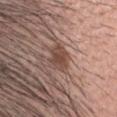biopsy status: imaged on a skin check; not biopsied | location: the head or neck | lighting: white-light | size: about 3.5 mm | automated metrics: a footprint of about 7 mm², an outline eccentricity of about 0.65 (0 = round, 1 = elongated), and a shape-asymmetry score of about 0.2 (0 = symmetric); a lesion color around L≈46 a*≈19 b*≈25 in CIELAB, roughly 10 lightness units darker than nearby skin, and a normalized border contrast of about 7.5 | acquisition: ~15 mm tile from a whole-body skin photo | subject: male, aged 33–37.